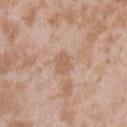<record>
<site>left upper arm</site>
<lesion_size>
  <long_diameter_mm_approx>2.5</long_diameter_mm_approx>
</lesion_size>
<image>
  <source>total-body photography crop</source>
  <field_of_view_mm>15</field_of_view_mm>
</image>
<patient>
  <sex>female</sex>
  <age_approx>25</age_approx>
</patient>
<lighting>white-light</lighting>
</record>